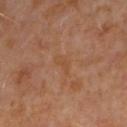Impression: The lesion was tiled from a total-body skin photograph and was not biopsied. Clinical summary: Approximately 2.5 mm at its widest. The tile uses cross-polarized illumination. The subject is a female aged around 50. The lesion is located on the left forearm. A lesion tile, about 15 mm wide, cut from a 3D total-body photograph.